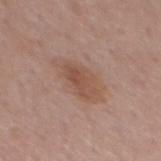Case summary:
– workup — total-body-photography surveillance lesion; no biopsy
– automated metrics — an outline eccentricity of about 0.8 (0 = round, 1 = elongated) and a shape-asymmetry score of about 0.25 (0 = symmetric); a peripheral color-asymmetry measure near 1
– lesion diameter — ~4 mm (longest diameter)
– acquisition — 15 mm crop, total-body photography
– anatomic site — the mid back
– illumination — white-light illumination
– patient — male, approximately 55 years of age